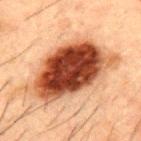Q: Is there a histopathology result?
A: total-body-photography surveillance lesion; no biopsy
Q: Lesion location?
A: the mid back
Q: Patient demographics?
A: male, approximately 50 years of age
Q: How large is the lesion?
A: ≈11 mm
Q: What is the imaging modality?
A: 15 mm crop, total-body photography
Q: How was the tile lit?
A: cross-polarized illumination
Q: What did automated image analysis measure?
A: a footprint of about 45 mm², an outline eccentricity of about 0.85 (0 = round, 1 = elongated), and two-axis asymmetry of about 0.2; a border-irregularity rating of about 3/10 and peripheral color asymmetry of about 3; a classifier nevus-likeness of about 95/100 and lesion-presence confidence of about 100/100